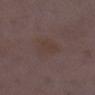Notes:
• follow-up — no biopsy performed (imaged during a skin exam)
• body site — the leg
• subject — female, approximately 30 years of age
• acquisition — total-body-photography crop, ~15 mm field of view